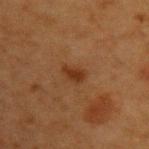<tbp_lesion>
<biopsy_status>not biopsied; imaged during a skin examination</biopsy_status>
<image>
  <source>total-body photography crop</source>
  <field_of_view_mm>15</field_of_view_mm>
</image>
<lighting>cross-polarized</lighting>
<lesion_size>
  <long_diameter_mm_approx>2.5</long_diameter_mm_approx>
</lesion_size>
<automated_metrics>
  <area_mm2_approx>3.5</area_mm2_approx>
  <eccentricity>0.8</eccentricity>
  <shape_asymmetry>0.25</shape_asymmetry>
  <cielab_L>30</cielab_L>
  <cielab_a>21</cielab_a>
  <cielab_b>31</cielab_b>
  <vs_skin_darker_L>8.0</vs_skin_darker_L>
  <border_irregularity_0_10>2.5</border_irregularity_0_10>
  <color_variation_0_10>1.0</color_variation_0_10>
</automated_metrics>
<patient>
  <sex>female</sex>
  <age_approx>40</age_approx>
</patient>
<site>left upper arm</site>
</tbp_lesion>